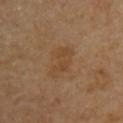workup = no biopsy performed (imaged during a skin exam)
patient = male, approximately 85 years of age or older
tile lighting = cross-polarized illumination
image source = total-body-photography crop, ~15 mm field of view
lesion diameter = ~4.5 mm (longest diameter)
location = the upper back